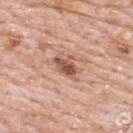biopsy status: total-body-photography surveillance lesion; no biopsy
patient: male, in their 80s
site: the upper back
imaging modality: ~15 mm tile from a whole-body skin photo
automated metrics: a footprint of about 5 mm² and an eccentricity of roughly 0.7; an average lesion color of about L≈54 a*≈24 b*≈29 (CIELAB), about 13 CIELAB-L* units darker than the surrounding skin, and a lesion-to-skin contrast of about 9 (normalized; higher = more distinct)
lighting: white-light illumination